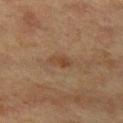No biopsy was performed on this lesion — it was imaged during a full skin examination and was not determined to be concerning. On the left thigh. A female patient, about 40 years old. A 15 mm close-up extracted from a 3D total-body photography capture.